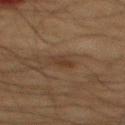Q: Is there a histopathology result?
A: imaged on a skin check; not biopsied
Q: Lesion location?
A: the mid back
Q: Illumination type?
A: cross-polarized
Q: What kind of image is this?
A: ~15 mm tile from a whole-body skin photo
Q: What is the lesion's diameter?
A: ≈2.5 mm
Q: Patient demographics?
A: male, aged 63–67
Q: What did automated image analysis measure?
A: a lesion area of about 3 mm², an outline eccentricity of about 0.8 (0 = round, 1 = elongated), and a symmetry-axis asymmetry near 0.3; a lesion color around L≈29 a*≈14 b*≈24 in CIELAB, a lesion–skin lightness drop of about 5, and a normalized lesion–skin contrast near 6; border irregularity of about 3 on a 0–10 scale and a within-lesion color-variation index near 2/10; a classifier nevus-likeness of about 5/100 and a detector confidence of about 100 out of 100 that the crop contains a lesion